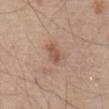The lesion was tiled from a total-body skin photograph and was not biopsied.
A 15 mm close-up tile from a total-body photography series done for melanoma screening.
On the front of the torso.
A male subject, approximately 80 years of age.
Measured at roughly 3 mm in maximum diameter.
Captured under white-light illumination.